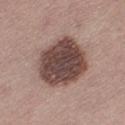Assessment: The lesion was photographed on a routine skin check and not biopsied; there is no pathology result. Context: Imaged with white-light lighting. About 6 mm across. Cropped from a whole-body photographic skin survey; the tile spans about 15 mm. On the leg. The patient is a female in their mid- to late 50s.